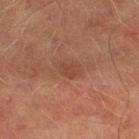tile lighting = cross-polarized
location = the right thigh
TBP lesion metrics = an area of roughly 4.5 mm², a shape eccentricity near 0.75, and a symmetry-axis asymmetry near 0.25
patient = male, aged 73–77
acquisition = total-body-photography crop, ~15 mm field of view